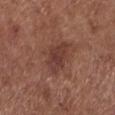Recorded during total-body skin imaging; not selected for excision or biopsy. Captured under white-light illumination. An algorithmic analysis of the crop reported a footprint of about 9 mm², an outline eccentricity of about 0.75 (0 = round, 1 = elongated), and a symmetry-axis asymmetry near 0.2. And it measured an average lesion color of about L≈38 a*≈22 b*≈24 (CIELAB) and a lesion–skin lightness drop of about 8. The software also gave a within-lesion color-variation index near 4/10 and radial color variation of about 1.5. The software also gave an automated nevus-likeness rating near 35 out of 100 and a lesion-detection confidence of about 100/100. The patient is a female aged 53 to 57. The lesion is on the left lower leg. A region of skin cropped from a whole-body photographic capture, roughly 15 mm wide. Longest diameter approximately 4.5 mm.